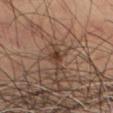workup: imaged on a skin check; not biopsied
imaging modality: ~15 mm crop, total-body skin-cancer survey
size: ≈1.5 mm
anatomic site: the left thigh
tile lighting: cross-polarized
patient: male, about 65 years old